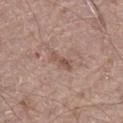notes: catalogued during a skin exam; not biopsied
subject: male, roughly 70 years of age
body site: the leg
tile lighting: white-light
size: about 3 mm
automated metrics: a lesion area of about 3.5 mm², an outline eccentricity of about 0.9 (0 = round, 1 = elongated), and a shape-asymmetry score of about 0.4 (0 = symmetric); a border-irregularity index near 4.5/10, a color-variation rating of about 0.5/10, and peripheral color asymmetry of about 0
imaging modality: ~15 mm crop, total-body skin-cancer survey